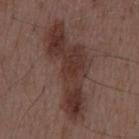The lesion was photographed on a routine skin check and not biopsied; there is no pathology result. Imaged with white-light lighting. A close-up tile cropped from a whole-body skin photograph, about 15 mm across. About 12 mm across. The lesion-visualizer software estimated a lesion color around L≈34 a*≈18 b*≈21 in CIELAB and a lesion-to-skin contrast of about 9 (normalized; higher = more distinct). It also reported a border-irregularity index near 7/10, internal color variation of about 4 on a 0–10 scale, and a peripheral color-asymmetry measure near 1.5. The lesion is located on the mid back. A male subject approximately 50 years of age.